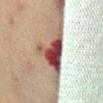Recorded during total-body skin imaging; not selected for excision or biopsy.
From the front of the torso.
A female patient aged 38 to 42.
Approximately 7.5 mm at its widest.
Imaged with cross-polarized lighting.
A 15 mm close-up extracted from a 3D total-body photography capture.